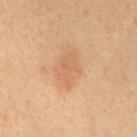Part of a total-body skin-imaging series; this lesion was reviewed on a skin check and was not flagged for biopsy. On the mid back. Measured at roughly 4.5 mm in maximum diameter. Imaged with cross-polarized lighting. The lesion-visualizer software estimated a lesion color around L≈46 a*≈16 b*≈29 in CIELAB, about 5 CIELAB-L* units darker than the surrounding skin, and a normalized border contrast of about 4.5. It also reported border irregularity of about 2 on a 0–10 scale, a within-lesion color-variation index near 2/10, and radial color variation of about 0.5. A lesion tile, about 15 mm wide, cut from a 3D total-body photograph. A female patient aged approximately 80.